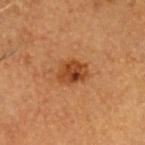biopsy status = no biopsy performed (imaged during a skin exam)
patient = male, in their mid-80s or older
lighting = cross-polarized
location = the head or neck
TBP lesion metrics = a lesion–skin lightness drop of about 10
image source = ~15 mm tile from a whole-body skin photo